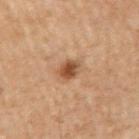Q: Was this lesion biopsied?
A: imaged on a skin check; not biopsied
Q: How was the tile lit?
A: cross-polarized
Q: What is the anatomic site?
A: the right upper arm
Q: How was this image acquired?
A: 15 mm crop, total-body photography
Q: Patient demographics?
A: male, aged approximately 70
Q: Lesion size?
A: ≈2.5 mm